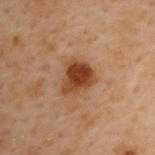No biopsy was performed on this lesion — it was imaged during a full skin examination and was not determined to be concerning.
An algorithmic analysis of the crop reported a footprint of about 9.5 mm² and two-axis asymmetry of about 0.35. The software also gave an average lesion color of about L≈37 a*≈21 b*≈31 (CIELAB), roughly 12 lightness units darker than nearby skin, and a normalized lesion–skin contrast near 10.5. And it measured a classifier nevus-likeness of about 95/100 and a detector confidence of about 100 out of 100 that the crop contains a lesion.
The lesion is on the upper back.
A 15 mm close-up tile from a total-body photography series done for melanoma screening.
About 4 mm across.
Imaged with cross-polarized lighting.
A female subject, approximately 60 years of age.